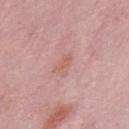Q: Is there a histopathology result?
A: no biopsy performed (imaged during a skin exam)
Q: What kind of image is this?
A: total-body-photography crop, ~15 mm field of view
Q: Automated lesion metrics?
A: a lesion color around L≈60 a*≈24 b*≈26 in CIELAB, about 6 CIELAB-L* units darker than the surrounding skin, and a normalized lesion–skin contrast near 5; a color-variation rating of about 0.5/10 and a peripheral color-asymmetry measure near 0; an automated nevus-likeness rating near 0 out of 100 and lesion-presence confidence of about 100/100
Q: How large is the lesion?
A: ~3 mm (longest diameter)
Q: Patient demographics?
A: male, aged approximately 55
Q: What lighting was used for the tile?
A: white-light illumination
Q: Lesion location?
A: the back